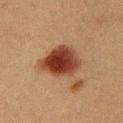| key | value |
|---|---|
| workup | no biopsy performed (imaged during a skin exam) |
| patient | male, in their 40s |
| TBP lesion metrics | an area of roughly 14 mm², an outline eccentricity of about 0.45 (0 = round, 1 = elongated), and a symmetry-axis asymmetry near 0.2; an average lesion color of about L≈33 a*≈23 b*≈27 (CIELAB), a lesion–skin lightness drop of about 15, and a normalized border contrast of about 13; a nevus-likeness score of about 100/100 and lesion-presence confidence of about 100/100 |
| imaging modality | total-body-photography crop, ~15 mm field of view |
| lesion size | ≈4.5 mm |
| tile lighting | cross-polarized illumination |
| location | the front of the torso |